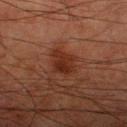Clinical impression: Imaged during a routine full-body skin examination; the lesion was not biopsied and no histopathology is available. Image and clinical context: Measured at roughly 3.5 mm in maximum diameter. On the right thigh. Cropped from a whole-body photographic skin survey; the tile spans about 15 mm. A male subject in their 80s. Captured under cross-polarized illumination.